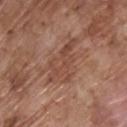Q: Was this lesion biopsied?
A: total-body-photography surveillance lesion; no biopsy